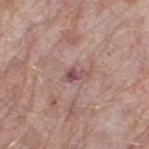Assessment:
No biopsy was performed on this lesion — it was imaged during a full skin examination and was not determined to be concerning.
Background:
The tile uses white-light illumination. Cropped from a total-body skin-imaging series; the visible field is about 15 mm. Approximately 2.5 mm at its widest. A female subject, aged around 70. Automated tile analysis of the lesion measured border irregularity of about 4.5 on a 0–10 scale and a color-variation rating of about 1.5/10. And it measured a nevus-likeness score of about 0/100. Located on the leg.